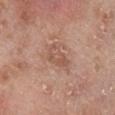Recorded during total-body skin imaging; not selected for excision or biopsy.
A 15 mm close-up tile from a total-body photography series done for melanoma screening.
A male subject, aged 73 to 77.
The lesion's longest dimension is about 3.5 mm.
Captured under white-light illumination.
On the right lower leg.